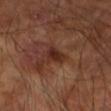workup = total-body-photography surveillance lesion; no biopsy | location = the right upper arm | subject = male, roughly 70 years of age | imaging modality = 15 mm crop, total-body photography | lighting = cross-polarized | diameter = about 2.5 mm.